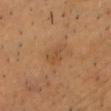<tbp_lesion>
  <site>head or neck</site>
  <patient>
    <sex>male</sex>
    <age_approx>60</age_approx>
  </patient>
  <lighting>cross-polarized</lighting>
  <automated_metrics>
    <area_mm2_approx>4.5</area_mm2_approx>
  </automated_metrics>
  <image>
    <source>total-body photography crop</source>
    <field_of_view_mm>15</field_of_view_mm>
  </image>
</tbp_lesion>